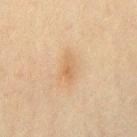follow-up: total-body-photography surveillance lesion; no biopsy | body site: the front of the torso | lighting: cross-polarized illumination | lesion size: about 3.5 mm | patient: male, aged 43–47 | image: ~15 mm tile from a whole-body skin photo.